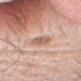| field | value |
|---|---|
| follow-up | no biopsy performed (imaged during a skin exam) |
| acquisition | 15 mm crop, total-body photography |
| location | the head or neck |
| diameter | ≈3 mm |
| patient | male, aged 33–37 |
| illumination | white-light illumination |